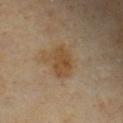Case summary:
* biopsy status · no biopsy performed (imaged during a skin exam)
* image source · ~15 mm crop, total-body skin-cancer survey
* body site · the chest
* tile lighting · cross-polarized
* automated lesion analysis · an average lesion color of about L≈47 a*≈16 b*≈34 (CIELAB); lesion-presence confidence of about 100/100
* lesion size · ≈4 mm
* subject · male, aged around 40